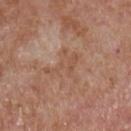workup: imaged on a skin check; not biopsied
image-analysis metrics: a lesion color around L≈52 a*≈20 b*≈30 in CIELAB, roughly 5 lightness units darker than nearby skin, and a normalized border contrast of about 4.5; an automated nevus-likeness rating near 0 out of 100 and lesion-presence confidence of about 100/100
acquisition: 15 mm crop, total-body photography
body site: the chest
patient: male, about 65 years old